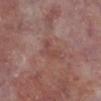{
  "automated_metrics": {
    "area_mm2_approx": 4.5,
    "eccentricity": 0.75,
    "shape_asymmetry": 0.5,
    "cielab_L": 46,
    "cielab_a": 23,
    "cielab_b": 23,
    "vs_skin_darker_L": 6.0,
    "vs_skin_contrast_norm": 5.0
  },
  "lighting": "white-light",
  "patient": {
    "sex": "male",
    "age_approx": 75
  },
  "site": "right lower leg",
  "image": {
    "source": "total-body photography crop",
    "field_of_view_mm": 15
  },
  "lesion_size": {
    "long_diameter_mm_approx": 3.0
  }
}